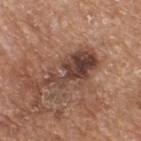No biopsy was performed on this lesion — it was imaged during a full skin examination and was not determined to be concerning.
Automated image analysis of the tile measured an area of roughly 14 mm², an outline eccentricity of about 0.95 (0 = round, 1 = elongated), and two-axis asymmetry of about 0.6. It also reported an average lesion color of about L≈41 a*≈20 b*≈25 (CIELAB). The software also gave border irregularity of about 9.5 on a 0–10 scale, internal color variation of about 5.5 on a 0–10 scale, and a peripheral color-asymmetry measure near 1.5. The analysis additionally found a classifier nevus-likeness of about 0/100 and lesion-presence confidence of about 100/100.
On the upper back.
A male patient, aged 63 to 67.
A close-up tile cropped from a whole-body skin photograph, about 15 mm across.